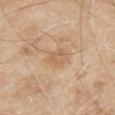| feature | finding |
|---|---|
| notes | total-body-photography surveillance lesion; no biopsy |
| subject | male, approximately 80 years of age |
| image source | ~15 mm crop, total-body skin-cancer survey |
| anatomic site | the back |
| size | ~3 mm (longest diameter) |
| tile lighting | white-light |